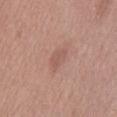Assessment:
The lesion was photographed on a routine skin check and not biopsied; there is no pathology result.
Acquisition and patient details:
The tile uses white-light illumination. A lesion tile, about 15 mm wide, cut from a 3D total-body photograph. Longest diameter approximately 3.5 mm. A male patient aged around 55.